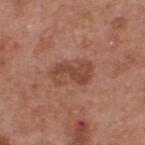notes — imaged on a skin check; not biopsied
size — ≈5 mm
imaging modality — 15 mm crop, total-body photography
site — the upper back
subject — male, aged 53–57